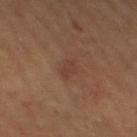Findings:
* follow-up · total-body-photography surveillance lesion; no biopsy
* image · ~15 mm crop, total-body skin-cancer survey
* TBP lesion metrics · internal color variation of about 0.5 on a 0–10 scale and peripheral color asymmetry of about 0; a nevus-likeness score of about 5/100 and a lesion-detection confidence of about 100/100
* site · the left thigh
* lighting · cross-polarized illumination
* subject · female, approximately 60 years of age
* lesion diameter · about 2.5 mm